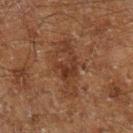Case summary:
– workup: total-body-photography surveillance lesion; no biopsy
– lesion diameter: ≈6.5 mm
– lighting: cross-polarized illumination
– anatomic site: the left lower leg
– acquisition: 15 mm crop, total-body photography
– patient: male, aged 58 to 62